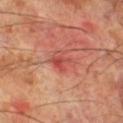Q: How was the tile lit?
A: cross-polarized illumination
Q: Lesion size?
A: about 2.5 mm
Q: How was this image acquired?
A: 15 mm crop, total-body photography
Q: Patient demographics?
A: male, roughly 70 years of age
Q: What is the anatomic site?
A: the right lower leg